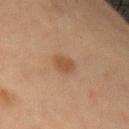Image and clinical context:
The patient is a male about 65 years old. A 15 mm close-up extracted from a 3D total-body photography capture. From the arm.